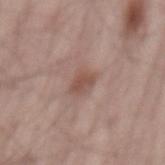Case summary:
• workup · imaged on a skin check; not biopsied
• image-analysis metrics · a mean CIELAB color near L≈51 a*≈19 b*≈24 and a normalized lesion–skin contrast near 6.5; a classifier nevus-likeness of about 15/100
• subject · male, aged 63 to 67
• lighting · white-light illumination
• size · ~2.5 mm (longest diameter)
• location · the back
• image source · 15 mm crop, total-body photography